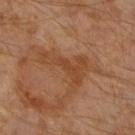Captured during whole-body skin photography for melanoma surveillance; the lesion was not biopsied. Measured at roughly 6 mm in maximum diameter. The lesion is on the right lower leg. A male patient, in their 60s. The total-body-photography lesion software estimated a lesion area of about 13 mm² and a shape eccentricity near 0.8. The analysis additionally found border irregularity of about 9.5 on a 0–10 scale, a within-lesion color-variation index near 2/10, and radial color variation of about 0.5. The tile uses cross-polarized illumination. A 15 mm close-up tile from a total-body photography series done for melanoma screening.